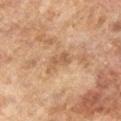<lesion>
<biopsy_status>not biopsied; imaged during a skin examination</biopsy_status>
<automated_metrics>
  <vs_skin_darker_L>8.0</vs_skin_darker_L>
  <vs_skin_contrast_norm>5.5</vs_skin_contrast_norm>
</automated_metrics>
<image>
  <source>total-body photography crop</source>
  <field_of_view_mm>15</field_of_view_mm>
</image>
<lesion_size>
  <long_diameter_mm_approx>3.0</long_diameter_mm_approx>
</lesion_size>
<patient>
  <sex>female</sex>
  <age_approx>60</age_approx>
</patient>
<site>left lower leg</site>
<lighting>cross-polarized</lighting>
</lesion>